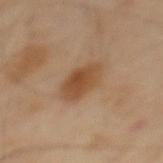Part of a total-body skin-imaging series; this lesion was reviewed on a skin check and was not flagged for biopsy.
A male patient, aged 38 to 42.
On the back.
The recorded lesion diameter is about 4.5 mm.
Cropped from a whole-body photographic skin survey; the tile spans about 15 mm.
This is a cross-polarized tile.